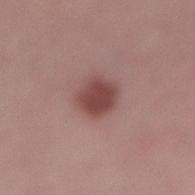Captured during whole-body skin photography for melanoma surveillance; the lesion was not biopsied.
Imaged with white-light lighting.
The lesion-visualizer software estimated an average lesion color of about L≈45 a*≈23 b*≈21 (CIELAB), roughly 12 lightness units darker than nearby skin, and a normalized lesion–skin contrast near 9.5. The software also gave border irregularity of about 1.5 on a 0–10 scale, internal color variation of about 2 on a 0–10 scale, and peripheral color asymmetry of about 0.5.
Cropped from a total-body skin-imaging series; the visible field is about 15 mm.
The lesion is located on the back.
A female patient, in their mid-50s.
Longest diameter approximately 3.5 mm.